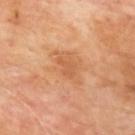biopsy status = no biopsy performed (imaged during a skin exam) | subject = male, aged 63 to 67 | illumination = cross-polarized illumination | body site = the back | imaging modality = ~15 mm tile from a whole-body skin photo | automated metrics = an area of roughly 3.5 mm² and two-axis asymmetry of about 0.4; an average lesion color of about L≈56 a*≈25 b*≈38 (CIELAB) and about 7 CIELAB-L* units darker than the surrounding skin; a color-variation rating of about 1.5/10; an automated nevus-likeness rating near 0 out of 100 and a lesion-detection confidence of about 100/100 | lesion diameter = ~2.5 mm (longest diameter).